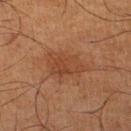Recorded during total-body skin imaging; not selected for excision or biopsy.
A male subject, in their mid-60s.
Automated image analysis of the tile measured a mean CIELAB color near L≈35 a*≈20 b*≈28 and a normalized border contrast of about 5.5. It also reported a border-irregularity index near 3.5/10, a within-lesion color-variation index near 2.5/10, and a peripheral color-asymmetry measure near 1.
A lesion tile, about 15 mm wide, cut from a 3D total-body photograph.
Measured at roughly 4.5 mm in maximum diameter.
The tile uses cross-polarized illumination.
Located on the right lower leg.